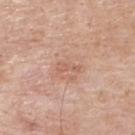Clinical impression:
Part of a total-body skin-imaging series; this lesion was reviewed on a skin check and was not flagged for biopsy.
Image and clinical context:
A 15 mm close-up extracted from a 3D total-body photography capture. Imaged with white-light lighting. The lesion's longest dimension is about 2.5 mm. The lesion is on the upper back. The total-body-photography lesion software estimated an average lesion color of about L≈60 a*≈22 b*≈29 (CIELAB), about 7 CIELAB-L* units darker than the surrounding skin, and a normalized lesion–skin contrast near 5. The software also gave a border-irregularity index near 5/10, internal color variation of about 0 on a 0–10 scale, and radial color variation of about 0. A male patient, aged approximately 65.